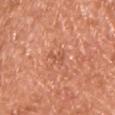{
  "automated_metrics": {
    "area_mm2_approx": 2.5,
    "eccentricity": 0.9,
    "shape_asymmetry": 0.65,
    "border_irregularity_0_10": 7.0,
    "color_variation_0_10": 0.0,
    "peripheral_color_asymmetry": 0.0
  },
  "site": "front of the torso",
  "patient": {
    "sex": "male",
    "age_approx": 55
  },
  "image": {
    "source": "total-body photography crop",
    "field_of_view_mm": 15
  },
  "lighting": "white-light"
}